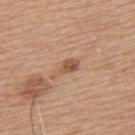Q: Was this lesion biopsied?
A: catalogued during a skin exam; not biopsied
Q: What is the anatomic site?
A: the upper back
Q: Lesion size?
A: about 3.5 mm
Q: Patient demographics?
A: male, in their mid- to late 60s
Q: What kind of image is this?
A: ~15 mm crop, total-body skin-cancer survey